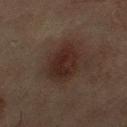Image and clinical context:
Located on the right thigh. A female patient about 55 years old. A lesion tile, about 15 mm wide, cut from a 3D total-body photograph.